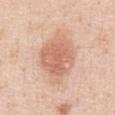This lesion was catalogued during total-body skin photography and was not selected for biopsy. Cropped from a whole-body photographic skin survey; the tile spans about 15 mm. A male subject, in their 50s. On the abdomen. Longest diameter approximately 6.5 mm.